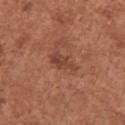Part of a total-body skin-imaging series; this lesion was reviewed on a skin check and was not flagged for biopsy. Located on the right upper arm. Cropped from a whole-body photographic skin survey; the tile spans about 15 mm. A female subject, aged 48 to 52.